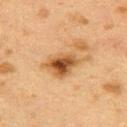Clinical impression:
Part of a total-body skin-imaging series; this lesion was reviewed on a skin check and was not flagged for biopsy.